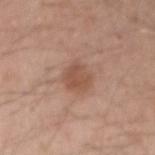  biopsy_status: not biopsied; imaged during a skin examination
  site: left forearm
  lesion_size:
    long_diameter_mm_approx: 3.0
  image:
    source: total-body photography crop
    field_of_view_mm: 15
  patient:
    sex: male
    age_approx: 50
  lighting: white-light